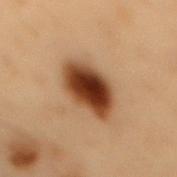Assessment: Recorded during total-body skin imaging; not selected for excision or biopsy. Image and clinical context: Cropped from a total-body skin-imaging series; the visible field is about 15 mm. Located on the mid back. The patient is a male roughly 55 years of age. The tile uses cross-polarized illumination. Measured at roughly 6 mm in maximum diameter.